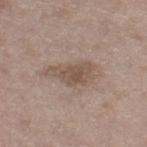- biopsy status · catalogued during a skin exam; not biopsied
- diameter · about 5.5 mm
- subject · male, aged 48–52
- site · the right thigh
- imaging modality · ~15 mm crop, total-body skin-cancer survey